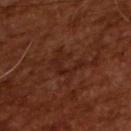  biopsy_status: not biopsied; imaged during a skin examination